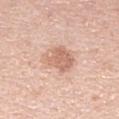biopsy status: no biopsy performed (imaged during a skin exam)
subject: female, in their 30s
tile lighting: white-light illumination
anatomic site: the right forearm
automated lesion analysis: a footprint of about 11 mm², an outline eccentricity of about 0.55 (0 = round, 1 = elongated), and two-axis asymmetry of about 0.2; about 10 CIELAB-L* units darker than the surrounding skin
imaging modality: total-body-photography crop, ~15 mm field of view
diameter: ≈4 mm